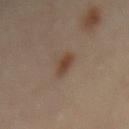Imaged during a routine full-body skin examination; the lesion was not biopsied and no histopathology is available. Located on the back. A female subject aged 58–62. About 3.5 mm across. The tile uses cross-polarized illumination. A roughly 15 mm field-of-view crop from a total-body skin photograph.